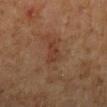No biopsy was performed on this lesion — it was imaged during a full skin examination and was not determined to be concerning.
The lesion is located on the right lower leg.
The recorded lesion diameter is about 3 mm.
The tile uses cross-polarized illumination.
A 15 mm crop from a total-body photograph taken for skin-cancer surveillance.
The total-body-photography lesion software estimated an area of roughly 4 mm². The analysis additionally found a border-irregularity index near 4/10, a color-variation rating of about 2/10, and radial color variation of about 1. The software also gave lesion-presence confidence of about 100/100.
A male subject, in their 60s.